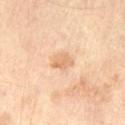The lesion was tiled from a total-body skin photograph and was not biopsied.
The lesion is on the leg.
A subject roughly 55 years of age.
A 15 mm crop from a total-body photograph taken for skin-cancer surveillance.